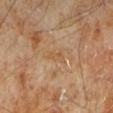The lesion is on the leg. Cropped from a total-body skin-imaging series; the visible field is about 15 mm. Imaged with cross-polarized lighting. Automated image analysis of the tile measured an area of roughly 2.5 mm², an eccentricity of roughly 0.9, and a symmetry-axis asymmetry near 0.4. The software also gave a color-variation rating of about 0/10 and radial color variation of about 0. The analysis additionally found lesion-presence confidence of about 95/100. The lesion's longest dimension is about 2.5 mm. A male subject about 70 years old.